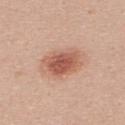No biopsy was performed on this lesion — it was imaged during a full skin examination and was not determined to be concerning. Located on the upper back. Automated tile analysis of the lesion measured an area of roughly 14 mm², an eccentricity of roughly 0.7, and a shape-asymmetry score of about 0.15 (0 = symmetric). The analysis additionally found about 12 CIELAB-L* units darker than the surrounding skin and a normalized lesion–skin contrast near 8. The analysis additionally found a color-variation rating of about 5/10 and radial color variation of about 1. Captured under white-light illumination. A female subject about 20 years old. A close-up tile cropped from a whole-body skin photograph, about 15 mm across.